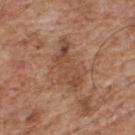<tbp_lesion>
  <biopsy_status>not biopsied; imaged during a skin examination</biopsy_status>
  <image>
    <source>total-body photography crop</source>
    <field_of_view_mm>15</field_of_view_mm>
  </image>
  <patient>
    <sex>male</sex>
    <age_approx>65</age_approx>
  </patient>
  <site>upper back</site>
  <lesion_size>
    <long_diameter_mm_approx>6.5</long_diameter_mm_approx>
  </lesion_size>
  <lighting>white-light</lighting>
</tbp_lesion>